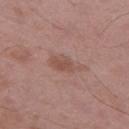Impression:
The lesion was photographed on a routine skin check and not biopsied; there is no pathology result.
Acquisition and patient details:
A 15 mm close-up tile from a total-body photography series done for melanoma screening. Located on the left thigh. The patient is a male roughly 50 years of age. Longest diameter approximately 4 mm.